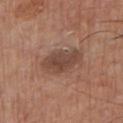workup — imaged on a skin check; not biopsied | location — the chest | acquisition — ~15 mm crop, total-body skin-cancer survey | patient — male, in their mid-60s.